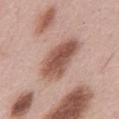• workup: catalogued during a skin exam; not biopsied
• tile lighting: white-light
• patient: male, in their mid- to late 50s
• anatomic site: the mid back
• lesion diameter: about 6 mm
• imaging modality: total-body-photography crop, ~15 mm field of view